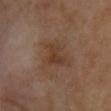biopsy status = total-body-photography surveillance lesion; no biopsy
body site = the chest
image = 15 mm crop, total-body photography
diameter = ~3 mm (longest diameter)
tile lighting = cross-polarized illumination
image-analysis metrics = a lesion color around L≈36 a*≈18 b*≈28 in CIELAB, a lesion–skin lightness drop of about 6, and a normalized border contrast of about 6; a border-irregularity index near 5/10
patient = female, aged 58–62